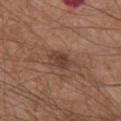follow-up: no biopsy performed (imaged during a skin exam) | location: the chest | automated metrics: a border-irregularity rating of about 1.5/10, internal color variation of about 3 on a 0–10 scale, and a peripheral color-asymmetry measure near 1; an automated nevus-likeness rating near 70 out of 100 and a detector confidence of about 100 out of 100 that the crop contains a lesion | image: ~15 mm crop, total-body skin-cancer survey | subject: male, about 65 years old.